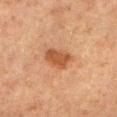notes: imaged on a skin check; not biopsied | imaging modality: total-body-photography crop, ~15 mm field of view | body site: the right lower leg | illumination: cross-polarized | subject: male, aged approximately 65.